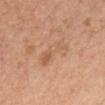biopsy_status: not biopsied; imaged during a skin examination
automated_metrics:
  area_mm2_approx: 8.0
  eccentricity: 0.9
  shape_asymmetry: 0.35
  cielab_L: 60
  cielab_a: 21
  cielab_b: 34
  border_irregularity_0_10: 4.5
  peripheral_color_asymmetry: 2.0
  nevus_likeness_0_100: 5
  lesion_detection_confidence_0_100: 100
lesion_size:
  long_diameter_mm_approx: 4.5
image:
  source: total-body photography crop
  field_of_view_mm: 15
patient:
  sex: female
  age_approx: 50
site: head or neck
lighting: white-light